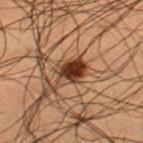The subject is a male in their 50s. The tile uses cross-polarized illumination. Longest diameter approximately 3.5 mm. The lesion-visualizer software estimated a shape eccentricity near 0.5 and a shape-asymmetry score of about 0.2 (0 = symmetric). The analysis additionally found border irregularity of about 2 on a 0–10 scale, internal color variation of about 7.5 on a 0–10 scale, and radial color variation of about 2.5. Located on the left thigh. Cropped from a whole-body photographic skin survey; the tile spans about 15 mm.